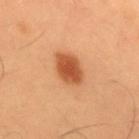biopsy_status: not biopsied; imaged during a skin examination
image:
  source: total-body photography crop
  field_of_view_mm: 15
lesion_size:
  long_diameter_mm_approx: 4.5
patient:
  sex: male
  age_approx: 55
site: mid back
automated_metrics:
  area_mm2_approx: 9.0
  eccentricity: 0.75
  shape_asymmetry: 0.15
  cielab_L: 52
  cielab_a: 28
  cielab_b: 40
  vs_skin_darker_L: 14.0
  vs_skin_contrast_norm: 9.5
  border_irregularity_0_10: 1.5
  color_variation_0_10: 3.0
  peripheral_color_asymmetry: 0.5
  lesion_detection_confidence_0_100: 100